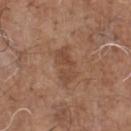Findings:
- workup: catalogued during a skin exam; not biopsied
- acquisition: ~15 mm tile from a whole-body skin photo
- diameter: ≈4.5 mm
- patient: male, aged around 70
- illumination: white-light
- automated metrics: lesion-presence confidence of about 100/100
- anatomic site: the chest